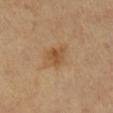<record>
  <biopsy_status>not biopsied; imaged during a skin examination</biopsy_status>
  <image>
    <source>total-body photography crop</source>
    <field_of_view_mm>15</field_of_view_mm>
  </image>
  <lighting>cross-polarized</lighting>
  <lesion_size>
    <long_diameter_mm_approx>2.5</long_diameter_mm_approx>
  </lesion_size>
  <patient>
    <sex>female</sex>
    <age_approx>70</age_approx>
  </patient>
  <site>right lower leg</site>
</record>